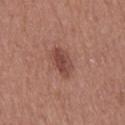Notes:
• biopsy status · total-body-photography surveillance lesion; no biopsy
• image source · ~15 mm tile from a whole-body skin photo
• illumination · white-light illumination
• patient · female, aged approximately 40
• diameter · ≈4 mm
• image-analysis metrics · a footprint of about 7 mm² and a shape-asymmetry score of about 0.2 (0 = symmetric)
• body site · the right thigh